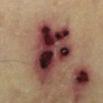| feature | finding |
|---|---|
| biopsy status | catalogued during a skin exam; not biopsied |
| automated lesion analysis | a lesion area of about 35 mm², an outline eccentricity of about 0.7 (0 = round, 1 = elongated), and two-axis asymmetry of about 0.35; a lesion color around L≈34 a*≈24 b*≈20 in CIELAB, a lesion–skin lightness drop of about 21, and a normalized lesion–skin contrast near 17.5; border irregularity of about 4 on a 0–10 scale, a within-lesion color-variation index near 10/10, and radial color variation of about 6.5 |
| anatomic site | the left lower leg |
| tile lighting | cross-polarized illumination |
| image | total-body-photography crop, ~15 mm field of view |
| size | about 8 mm |
| subject | about 60 years old |